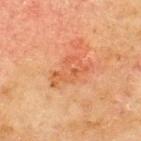The lesion was photographed on a routine skin check and not biopsied; there is no pathology result. The lesion is located on the upper back. A lesion tile, about 15 mm wide, cut from a 3D total-body photograph. A male patient in their 70s.